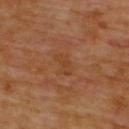workup: no biopsy performed (imaged during a skin exam)
image-analysis metrics: a mean CIELAB color near L≈40 a*≈22 b*≈34, a lesion–skin lightness drop of about 5, and a lesion-to-skin contrast of about 5 (normalized; higher = more distinct); a border-irregularity rating of about 3.5/10, internal color variation of about 0.5 on a 0–10 scale, and radial color variation of about 0
image source: ~15 mm crop, total-body skin-cancer survey
subject: male, aged 58 to 62
lesion diameter: ≈3 mm
tile lighting: cross-polarized
location: the upper back